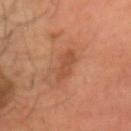Captured during whole-body skin photography for melanoma surveillance; the lesion was not biopsied. The lesion's longest dimension is about 4 mm. This image is a 15 mm lesion crop taken from a total-body photograph. The subject is a male aged 53–57. The lesion-visualizer software estimated an average lesion color of about L≈45 a*≈23 b*≈32 (CIELAB), a lesion–skin lightness drop of about 7, and a lesion-to-skin contrast of about 5.5 (normalized; higher = more distinct). It also reported a classifier nevus-likeness of about 0/100 and a lesion-detection confidence of about 100/100. Located on the head or neck.